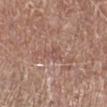Notes:
- anatomic site · the right lower leg
- subject · male, roughly 80 years of age
- image source · 15 mm crop, total-body photography